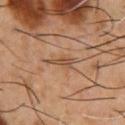Findings:
* notes: catalogued during a skin exam; not biopsied
* patient: male, aged 53 to 57
* size: ≈2.5 mm
* lighting: cross-polarized illumination
* image: total-body-photography crop, ~15 mm field of view
* site: the chest
* image-analysis metrics: a footprint of about 2.5 mm² and an outline eccentricity of about 0.9 (0 = round, 1 = elongated); a nevus-likeness score of about 0/100 and a detector confidence of about 55 out of 100 that the crop contains a lesion